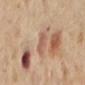Q: Is there a histopathology result?
A: imaged on a skin check; not biopsied
Q: Who is the patient?
A: female, about 55 years old
Q: What is the imaging modality?
A: ~15 mm crop, total-body skin-cancer survey
Q: How was the tile lit?
A: cross-polarized
Q: Where on the body is the lesion?
A: the mid back
Q: What is the lesion's diameter?
A: ~3 mm (longest diameter)